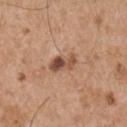Q: Is there a histopathology result?
A: imaged on a skin check; not biopsied
Q: How was the tile lit?
A: white-light
Q: Automated lesion metrics?
A: an average lesion color of about L≈50 a*≈21 b*≈30 (CIELAB) and a lesion-to-skin contrast of about 8.5 (normalized; higher = more distinct); a border-irregularity rating of about 3/10, a color-variation rating of about 9.5/10, and radial color variation of about 4; a nevus-likeness score of about 75/100 and lesion-presence confidence of about 100/100
Q: What kind of image is this?
A: total-body-photography crop, ~15 mm field of view
Q: Patient demographics?
A: male, approximately 55 years of age
Q: Where on the body is the lesion?
A: the right upper arm